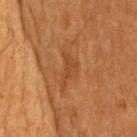biopsy status: imaged on a skin check; not biopsied | TBP lesion metrics: a mean CIELAB color near L≈36 a*≈21 b*≈32 and a lesion-to-skin contrast of about 5 (normalized; higher = more distinct) | body site: the head or neck | lesion diameter: about 4 mm | subject: male, about 75 years old | lighting: cross-polarized illumination | image source: total-body-photography crop, ~15 mm field of view.